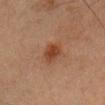Impression: Captured during whole-body skin photography for melanoma surveillance; the lesion was not biopsied. Context: An algorithmic analysis of the crop reported an average lesion color of about L≈37 a*≈22 b*≈30 (CIELAB) and about 9 CIELAB-L* units darker than the surrounding skin. The analysis additionally found a border-irregularity index near 2/10, a within-lesion color-variation index near 2/10, and peripheral color asymmetry of about 0.5. A 15 mm close-up extracted from a 3D total-body photography capture. Imaged with cross-polarized lighting. A female patient aged around 45. The lesion is on the head or neck.